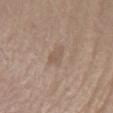| feature | finding |
|---|---|
| follow-up | no biopsy performed (imaged during a skin exam) |
| acquisition | ~15 mm crop, total-body skin-cancer survey |
| patient | male, about 65 years old |
| body site | the right forearm |